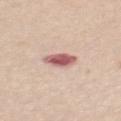biopsy_status: not biopsied; imaged during a skin examination
automated_metrics:
  area_mm2_approx: 6.0
  eccentricity: 0.65
  shape_asymmetry: 0.2
  border_irregularity_0_10: 2.0
  color_variation_0_10: 4.0
  peripheral_color_asymmetry: 1.0
site: mid back
lighting: white-light
image:
  source: total-body photography crop
  field_of_view_mm: 15
patient:
  sex: female
  age_approx: 55